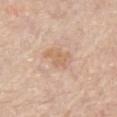Clinical impression: Captured during whole-body skin photography for melanoma surveillance; the lesion was not biopsied. Clinical summary: A roughly 15 mm field-of-view crop from a total-body skin photograph. Captured under white-light illumination. From the front of the torso. Longest diameter approximately 4 mm. The subject is a male roughly 75 years of age.